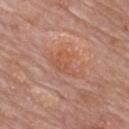biopsy status: total-body-photography surveillance lesion; no biopsy | body site: the chest | automated metrics: a footprint of about 8 mm² and a symmetry-axis asymmetry near 0.55; a border-irregularity index near 6/10, internal color variation of about 3 on a 0–10 scale, and peripheral color asymmetry of about 1; an automated nevus-likeness rating near 5 out of 100 | image source: 15 mm crop, total-body photography | lesion diameter: ~4.5 mm (longest diameter) | patient: male, about 60 years old.